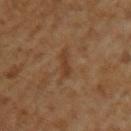Assessment:
Imaged during a routine full-body skin examination; the lesion was not biopsied and no histopathology is available.
Acquisition and patient details:
A male subject aged 58–62. Automated image analysis of the tile measured a lesion color around L≈34 a*≈18 b*≈29 in CIELAB, roughly 6 lightness units darker than nearby skin, and a lesion-to-skin contrast of about 6 (normalized; higher = more distinct). A lesion tile, about 15 mm wide, cut from a 3D total-body photograph. Measured at roughly 3 mm in maximum diameter. The tile uses cross-polarized illumination. The lesion is located on the left upper arm.